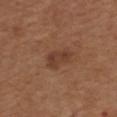follow-up: no biopsy performed (imaged during a skin exam)
acquisition: total-body-photography crop, ~15 mm field of view
subject: male, aged approximately 75
size: about 3.5 mm
location: the upper back